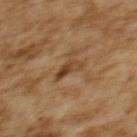Part of a total-body skin-imaging series; this lesion was reviewed on a skin check and was not flagged for biopsy.
About 3.5 mm across.
The subject is a female about 60 years old.
A 15 mm crop from a total-body photograph taken for skin-cancer surveillance.
An algorithmic analysis of the crop reported a lesion area of about 4.5 mm², an eccentricity of roughly 0.9, and a shape-asymmetry score of about 0.4 (0 = symmetric). It also reported a lesion color around L≈37 a*≈16 b*≈30 in CIELAB and a normalized lesion–skin contrast near 7.5. The software also gave a nevus-likeness score of about 15/100 and a lesion-detection confidence of about 95/100.
The lesion is on the upper back.
Imaged with cross-polarized lighting.